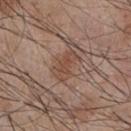{"biopsy_status": "not biopsied; imaged during a skin examination", "patient": {"sex": "male", "age_approx": 55}, "image": {"source": "total-body photography crop", "field_of_view_mm": 15}, "lighting": "white-light", "site": "chest", "automated_metrics": {"area_mm2_approx": 7.5, "eccentricity": 0.85, "shape_asymmetry": 0.35, "vs_skin_darker_L": 7.0, "vs_skin_contrast_norm": 6.0, "nevus_likeness_0_100": 10, "lesion_detection_confidence_0_100": 100}, "lesion_size": {"long_diameter_mm_approx": 4.0}}